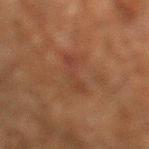Part of a total-body skin-imaging series; this lesion was reviewed on a skin check and was not flagged for biopsy.
A male patient, roughly 60 years of age.
This is a cross-polarized tile.
A region of skin cropped from a whole-body photographic capture, roughly 15 mm wide.
Located on the left lower leg.
The recorded lesion diameter is about 3.5 mm.